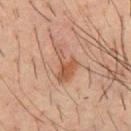The lesion was photographed on a routine skin check and not biopsied; there is no pathology result. Captured under cross-polarized illumination. An algorithmic analysis of the crop reported a mean CIELAB color near L≈50 a*≈20 b*≈30 and a normalized lesion–skin contrast near 7. And it measured border irregularity of about 7 on a 0–10 scale, a within-lesion color-variation index near 3.5/10, and a peripheral color-asymmetry measure near 1. A 15 mm close-up tile from a total-body photography series done for melanoma screening. On the front of the torso. The lesion's longest dimension is about 4.5 mm. The subject is a male in their mid-30s.